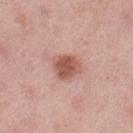notes = total-body-photography surveillance lesion; no biopsy | patient = female, aged 48–52 | size = ≈3 mm | image source = total-body-photography crop, ~15 mm field of view | illumination = white-light | automated lesion analysis = an area of roughly 7.5 mm², an eccentricity of roughly 0.4, and a symmetry-axis asymmetry near 0.2 | body site = the left thigh.